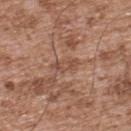Clinical impression: Captured during whole-body skin photography for melanoma surveillance; the lesion was not biopsied. Acquisition and patient details: A male patient aged 53–57. This image is a 15 mm lesion crop taken from a total-body photograph. Located on the back. The lesion-visualizer software estimated an area of roughly 3 mm², an eccentricity of roughly 0.9, and a shape-asymmetry score of about 0.45 (0 = symmetric). The analysis additionally found roughly 7 lightness units darker than nearby skin and a normalized lesion–skin contrast near 5.5. The software also gave border irregularity of about 4.5 on a 0–10 scale, internal color variation of about 0.5 on a 0–10 scale, and radial color variation of about 0. Captured under white-light illumination.